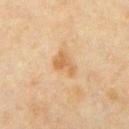Captured during whole-body skin photography for melanoma surveillance; the lesion was not biopsied. The tile uses cross-polarized illumination. The lesion's longest dimension is about 3.5 mm. A close-up tile cropped from a whole-body skin photograph, about 15 mm across. On the mid back. A male subject, about 60 years old.